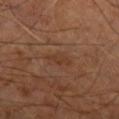| key | value |
|---|---|
| biopsy status | catalogued during a skin exam; not biopsied |
| image-analysis metrics | a lesion–skin lightness drop of about 5; a nevus-likeness score of about 0/100 and a detector confidence of about 100 out of 100 that the crop contains a lesion |
| diameter | ~3 mm (longest diameter) |
| tile lighting | cross-polarized |
| site | the right leg |
| imaging modality | total-body-photography crop, ~15 mm field of view |
| subject | male, in their 60s |